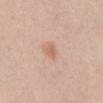biopsy status — imaged on a skin check; not biopsied | TBP lesion metrics — a footprint of about 3.5 mm², a shape eccentricity near 0.7, and a symmetry-axis asymmetry near 0.3; a within-lesion color-variation index near 1.5/10 and a peripheral color-asymmetry measure near 0.5 | illumination — white-light illumination | imaging modality — ~15 mm tile from a whole-body skin photo | diameter — ≈2.5 mm | patient — female, aged approximately 20 | site — the abdomen.